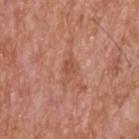Q: Is there a histopathology result?
A: no biopsy performed (imaged during a skin exam)
Q: How large is the lesion?
A: ≈3.5 mm
Q: What is the anatomic site?
A: the upper back
Q: How was the tile lit?
A: white-light illumination
Q: What is the imaging modality?
A: ~15 mm crop, total-body skin-cancer survey
Q: What are the patient's age and sex?
A: male, aged approximately 65